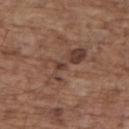Case summary:
- workup: imaged on a skin check; not biopsied